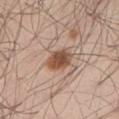biopsy_status: not biopsied; imaged during a skin examination
patient:
  sex: male
  age_approx: 55
lighting: white-light
automated_metrics:
  nevus_likeness_0_100: 100
  lesion_detection_confidence_0_100: 100
site: abdomen
image:
  source: total-body photography crop
  field_of_view_mm: 15